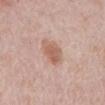Clinical impression: No biopsy was performed on this lesion — it was imaged during a full skin examination and was not determined to be concerning. Background: The tile uses white-light illumination. Measured at roughly 4 mm in maximum diameter. A male subject in their mid-70s. Cropped from a whole-body photographic skin survey; the tile spans about 15 mm. The lesion is located on the mid back.